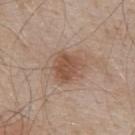size: ~3.5 mm (longest diameter); patient: male, in their mid-50s; lighting: white-light; site: the upper back; image: total-body-photography crop, ~15 mm field of view.